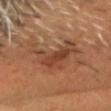| field | value |
|---|---|
| workup | no biopsy performed (imaged during a skin exam) |
| image | total-body-photography crop, ~15 mm field of view |
| illumination | cross-polarized |
| body site | the head or neck |
| lesion diameter | ≈8 mm |
| subject | male, aged around 55 |